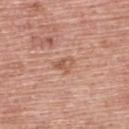biopsy status: catalogued during a skin exam; not biopsied
image: total-body-photography crop, ~15 mm field of view
lighting: white-light
automated lesion analysis: a lesion area of about 3.5 mm², an outline eccentricity of about 0.75 (0 = round, 1 = elongated), and a shape-asymmetry score of about 0.35 (0 = symmetric); about 8 CIELAB-L* units darker than the surrounding skin and a normalized border contrast of about 6
lesion size: about 2.5 mm
body site: the upper back
subject: male, about 60 years old